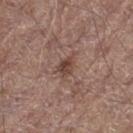Impression: Captured during whole-body skin photography for melanoma surveillance; the lesion was not biopsied. Image and clinical context: About 3.5 mm across. Captured under white-light illumination. The lesion is located on the left thigh. Automated tile analysis of the lesion measured a lesion area of about 4.5 mm², an outline eccentricity of about 0.8 (0 = round, 1 = elongated), and a shape-asymmetry score of about 0.3 (0 = symmetric). It also reported a lesion color around L≈44 a*≈18 b*≈24 in CIELAB, a lesion–skin lightness drop of about 9, and a lesion-to-skin contrast of about 7.5 (normalized; higher = more distinct). It also reported a border-irregularity rating of about 3.5/10, internal color variation of about 4 on a 0–10 scale, and peripheral color asymmetry of about 1.5. And it measured a nevus-likeness score of about 15/100 and a detector confidence of about 100 out of 100 that the crop contains a lesion. A lesion tile, about 15 mm wide, cut from a 3D total-body photograph. The subject is a male roughly 70 years of age.